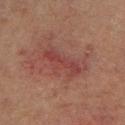Imaged during a routine full-body skin examination; the lesion was not biopsied and no histopathology is available.
The lesion is located on the leg.
The subject is a male aged 63 to 67.
Measured at roughly 5.5 mm in maximum diameter.
The tile uses cross-polarized illumination.
This image is a 15 mm lesion crop taken from a total-body photograph.